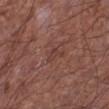Recorded during total-body skin imaging; not selected for excision or biopsy.
The subject is a male aged 63 to 67.
Imaged with white-light lighting.
On the left lower leg.
Approximately 2.5 mm at its widest.
A lesion tile, about 15 mm wide, cut from a 3D total-body photograph.
An algorithmic analysis of the crop reported a footprint of about 2.5 mm² and an eccentricity of roughly 0.8. The software also gave about 5 CIELAB-L* units darker than the surrounding skin and a lesion-to-skin contrast of about 5 (normalized; higher = more distinct). And it measured a border-irregularity index near 6.5/10, a color-variation rating of about 0/10, and peripheral color asymmetry of about 0.